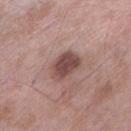Recorded during total-body skin imaging; not selected for excision or biopsy. A male subject about 55 years old. From the left lower leg. The lesion-visualizer software estimated an area of roughly 9 mm² and two-axis asymmetry of about 0.2. A roughly 15 mm field-of-view crop from a total-body skin photograph. The tile uses white-light illumination.